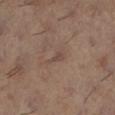This lesion was catalogued during total-body skin photography and was not selected for biopsy. The tile uses cross-polarized illumination. Automated image analysis of the tile measured a lesion area of about 2.5 mm² and an outline eccentricity of about 0.9 (0 = round, 1 = elongated). The analysis additionally found border irregularity of about 5.5 on a 0–10 scale and a color-variation rating of about 0/10. On the left lower leg. This image is a 15 mm lesion crop taken from a total-body photograph. Longest diameter approximately 2.5 mm. The patient is a female aged 58–62.